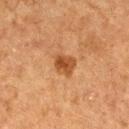biopsy status: catalogued during a skin exam; not biopsied | lighting: cross-polarized | TBP lesion metrics: a lesion area of about 5 mm² and two-axis asymmetry of about 0.25; a mean CIELAB color near L≈40 a*≈22 b*≈34, roughly 10 lightness units darker than nearby skin, and a normalized lesion–skin contrast near 8.5 | diameter: about 2.5 mm | subject: male, aged 73 to 77 | image source: ~15 mm crop, total-body skin-cancer survey | location: the leg.